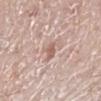Clinical impression:
Part of a total-body skin-imaging series; this lesion was reviewed on a skin check and was not flagged for biopsy.
Acquisition and patient details:
A close-up tile cropped from a whole-body skin photograph, about 15 mm across. Automated image analysis of the tile measured a lesion color around L≈60 a*≈19 b*≈25 in CIELAB and a lesion-to-skin contrast of about 6.5 (normalized; higher = more distinct). The software also gave a border-irregularity index near 4/10, a color-variation rating of about 1.5/10, and a peripheral color-asymmetry measure near 0.5. It also reported a classifier nevus-likeness of about 5/100 and lesion-presence confidence of about 95/100. The lesion is located on the right leg. About 2.5 mm across. A male subject aged 68–72.